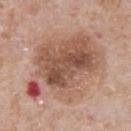notes: total-body-photography surveillance lesion; no biopsy | image: total-body-photography crop, ~15 mm field of view | anatomic site: the abdomen | subject: male, aged around 60 | tile lighting: white-light illumination | lesion size: ~11 mm (longest diameter).